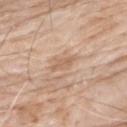The lesion was tiled from a total-body skin photograph and was not biopsied.
On the upper back.
A 15 mm close-up tile from a total-body photography series done for melanoma screening.
A male patient roughly 75 years of age.
This is a white-light tile.
The lesion's longest dimension is about 3.5 mm.